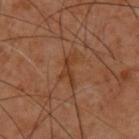The lesion was tiled from a total-body skin photograph and was not biopsied. On the back. The lesion's longest dimension is about 4 mm. The subject is a male in their 60s. A 15 mm crop from a total-body photograph taken for skin-cancer surveillance. This is a cross-polarized tile.